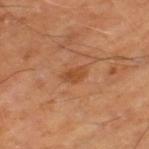Impression: Captured during whole-body skin photography for melanoma surveillance; the lesion was not biopsied. Clinical summary: The tile uses cross-polarized illumination. The patient is aged 63–67. The lesion is on the left thigh. The total-body-photography lesion software estimated a footprint of about 3.5 mm² and an eccentricity of roughly 0.85. It also reported a border-irregularity index near 2.5/10. A 15 mm crop from a total-body photograph taken for skin-cancer surveillance.